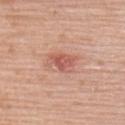No biopsy was performed on this lesion — it was imaged during a full skin examination and was not determined to be concerning. The subject is a female approximately 60 years of age. About 3.5 mm across. The lesion is located on the upper back. This is a white-light tile. The lesion-visualizer software estimated a footprint of about 7 mm², an eccentricity of roughly 0.75, and a shape-asymmetry score of about 0.35 (0 = symmetric). The software also gave a lesion-to-skin contrast of about 6.5 (normalized; higher = more distinct). The analysis additionally found border irregularity of about 3.5 on a 0–10 scale and a peripheral color-asymmetry measure near 1.5. It also reported a detector confidence of about 100 out of 100 that the crop contains a lesion. A 15 mm close-up tile from a total-body photography series done for melanoma screening.